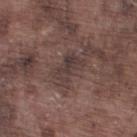The lesion was tiled from a total-body skin photograph and was not biopsied.